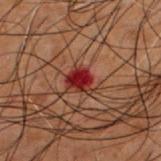Assessment:
Part of a total-body skin-imaging series; this lesion was reviewed on a skin check and was not flagged for biopsy.
Acquisition and patient details:
A male patient about 50 years old. Longest diameter approximately 3 mm. A roughly 15 mm field-of-view crop from a total-body skin photograph. From the upper back.